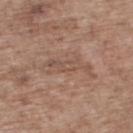<case>
<biopsy_status>not biopsied; imaged during a skin examination</biopsy_status>
<lighting>white-light</lighting>
<site>upper back</site>
<automated_metrics>
  <cielab_L>51</cielab_L>
  <cielab_a>18</cielab_a>
  <cielab_b>26</cielab_b>
  <vs_skin_darker_L>6.0</vs_skin_darker_L>
  <vs_skin_contrast_norm>4.5</vs_skin_contrast_norm>
</automated_metrics>
<image>
  <source>total-body photography crop</source>
  <field_of_view_mm>15</field_of_view_mm>
</image>
<patient>
  <sex>male</sex>
  <age_approx>70</age_approx>
</patient>
</case>